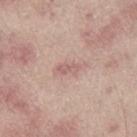Assessment:
Captured during whole-body skin photography for melanoma surveillance; the lesion was not biopsied.
Context:
The lesion's longest dimension is about 3 mm. The lesion is located on the leg. A roughly 15 mm field-of-view crop from a total-body skin photograph. Captured under white-light illumination. A male patient, aged 63 to 67.